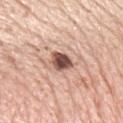biopsy status: total-body-photography surveillance lesion; no biopsy | size: ~3 mm (longest diameter) | location: the arm | acquisition: ~15 mm tile from a whole-body skin photo | illumination: white-light | subject: female, aged 38–42 | automated metrics: an area of roughly 5.5 mm² and an outline eccentricity of about 0.7 (0 = round, 1 = elongated); a lesion-to-skin contrast of about 12.5 (normalized; higher = more distinct); a classifier nevus-likeness of about 95/100.